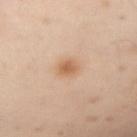Part of a total-body skin-imaging series; this lesion was reviewed on a skin check and was not flagged for biopsy. Located on the right forearm. Approximately 2.5 mm at its widest. Automated tile analysis of the lesion measured a border-irregularity rating of about 1.5/10 and peripheral color asymmetry of about 0.5. A male patient in their mid- to late 50s. A 15 mm close-up tile from a total-body photography series done for melanoma screening. Imaged with cross-polarized lighting.